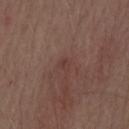Clinical impression: No biopsy was performed on this lesion — it was imaged during a full skin examination and was not determined to be concerning. Clinical summary: A male subject, about 70 years old. The tile uses white-light illumination. Measured at roughly 1.5 mm in maximum diameter. On the mid back. A lesion tile, about 15 mm wide, cut from a 3D total-body photograph.